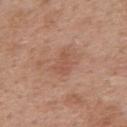| feature | finding |
|---|---|
| workup | no biopsy performed (imaged during a skin exam) |
| image source | 15 mm crop, total-body photography |
| patient | female, aged approximately 40 |
| location | the upper back |
| diameter | ≈3.5 mm |
| automated metrics | a nevus-likeness score of about 0/100 and lesion-presence confidence of about 100/100 |
| tile lighting | white-light illumination |